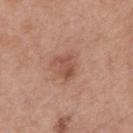workup=catalogued during a skin exam; not biopsied | tile lighting=white-light illumination | body site=the upper back | automated metrics=an area of roughly 5 mm², a shape eccentricity near 0.7, and a symmetry-axis asymmetry near 0.25; a mean CIELAB color near L≈51 a*≈24 b*≈28 and a normalized lesion–skin contrast near 6.5; peripheral color asymmetry of about 1; a classifier nevus-likeness of about 20/100 and lesion-presence confidence of about 100/100 | acquisition=15 mm crop, total-body photography | size=~3 mm (longest diameter) | patient=male, about 55 years old.